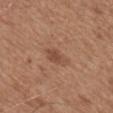workup = no biopsy performed (imaged during a skin exam) | subject = male, approximately 65 years of age | image source = 15 mm crop, total-body photography | body site = the mid back | tile lighting = white-light.